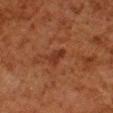follow-up: no biopsy performed (imaged during a skin exam) | lesion size: about 2.5 mm | tile lighting: cross-polarized | site: the right lower leg | automated lesion analysis: an area of roughly 3 mm², an outline eccentricity of about 0.85 (0 = round, 1 = elongated), and a shape-asymmetry score of about 0.3 (0 = symmetric); a nevus-likeness score of about 30/100 and a detector confidence of about 100 out of 100 that the crop contains a lesion | subject: male, aged 78–82 | acquisition: 15 mm crop, total-body photography.